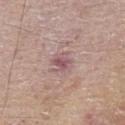biopsy_status: not biopsied; imaged during a skin examination
patient:
  sex: male
  age_approx: 85+
site: abdomen
lesion_size:
  long_diameter_mm_approx: 2.5
image:
  source: total-body photography crop
  field_of_view_mm: 15
lighting: white-light